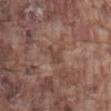notes: catalogued during a skin exam; not biopsied | site: the abdomen | illumination: white-light | image source: ~15 mm tile from a whole-body skin photo | size: about 2.5 mm | patient: male, about 75 years old.